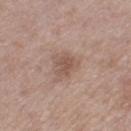| field | value |
|---|---|
| biopsy status | imaged on a skin check; not biopsied |
| lesion diameter | about 3 mm |
| body site | the left lower leg |
| subject | female, in their mid- to late 50s |
| image source | total-body-photography crop, ~15 mm field of view |
| lighting | white-light illumination |